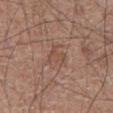Impression:
This lesion was catalogued during total-body skin photography and was not selected for biopsy.
Clinical summary:
A 15 mm crop from a total-body photograph taken for skin-cancer surveillance. The lesion is on the front of the torso. A male patient roughly 75 years of age.